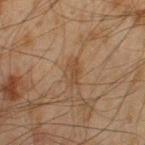<lesion>
<biopsy_status>not biopsied; imaged during a skin examination</biopsy_status>
<automated_metrics>
  <color_variation_0_10>2.0</color_variation_0_10>
  <peripheral_color_asymmetry>0.5</peripheral_color_asymmetry>
  <lesion_detection_confidence_0_100>100</lesion_detection_confidence_0_100>
</automated_metrics>
<patient>
  <sex>male</sex>
  <age_approx>45</age_approx>
</patient>
<site>left thigh</site>
<image>
  <source>total-body photography crop</source>
  <field_of_view_mm>15</field_of_view_mm>
</image>
<lighting>cross-polarized</lighting>
<lesion_size>
  <long_diameter_mm_approx>3.0</long_diameter_mm_approx>
</lesion_size>
</lesion>